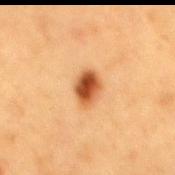Part of a total-body skin-imaging series; this lesion was reviewed on a skin check and was not flagged for biopsy.
A region of skin cropped from a whole-body photographic capture, roughly 15 mm wide.
The patient is a female approximately 40 years of age.
From the mid back.